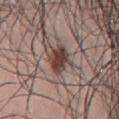<record>
  <biopsy_status>not biopsied; imaged during a skin examination</biopsy_status>
  <image>
    <source>total-body photography crop</source>
    <field_of_view_mm>15</field_of_view_mm>
  </image>
  <patient>
    <sex>male</sex>
    <age_approx>70</age_approx>
  </patient>
  <site>front of the torso</site>
  <lighting>white-light</lighting>
  <lesion_size>
    <long_diameter_mm_approx>3.5</long_diameter_mm_approx>
  </lesion_size>
</record>